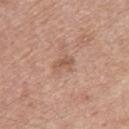biopsy status: imaged on a skin check; not biopsied
lesion size: ~2.5 mm (longest diameter)
location: the upper back
TBP lesion metrics: a mean CIELAB color near L≈56 a*≈20 b*≈29 and roughly 8 lightness units darker than nearby skin; a nevus-likeness score of about 0/100 and a detector confidence of about 100 out of 100 that the crop contains a lesion
image source: 15 mm crop, total-body photography
subject: male, aged 68–72